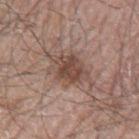Assessment: Recorded during total-body skin imaging; not selected for excision or biopsy. Clinical summary: Measured at roughly 6.5 mm in maximum diameter. Located on the left upper arm. The lesion-visualizer software estimated an area of roughly 14 mm², an outline eccentricity of about 0.75 (0 = round, 1 = elongated), and a symmetry-axis asymmetry near 0.25. And it measured a mean CIELAB color near L≈48 a*≈17 b*≈24, roughly 10 lightness units darker than nearby skin, and a normalized lesion–skin contrast near 7.5. Cropped from a total-body skin-imaging series; the visible field is about 15 mm. A male patient, aged 63 to 67. This is a white-light tile.